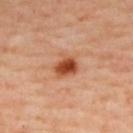workup: imaged on a skin check; not biopsied | patient: male, aged 53 to 57 | body site: the back | TBP lesion metrics: a normalized lesion–skin contrast near 10.5; border irregularity of about 2 on a 0–10 scale, a within-lesion color-variation index near 5/10, and a peripheral color-asymmetry measure near 1.5; a classifier nevus-likeness of about 100/100 and a detector confidence of about 100 out of 100 that the crop contains a lesion | size: ~3 mm (longest diameter) | tile lighting: cross-polarized | image: 15 mm crop, total-body photography.